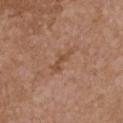Assessment: The lesion was tiled from a total-body skin photograph and was not biopsied. Clinical summary: Longest diameter approximately 3.5 mm. From the chest. A female patient, in their mid- to late 60s. The total-body-photography lesion software estimated a lesion area of about 3.5 mm² and a shape-asymmetry score of about 0.4 (0 = symmetric). The analysis additionally found a mean CIELAB color near L≈49 a*≈21 b*≈31, about 7 CIELAB-L* units darker than the surrounding skin, and a normalized border contrast of about 6. The software also gave a nevus-likeness score of about 0/100. A close-up tile cropped from a whole-body skin photograph, about 15 mm across.